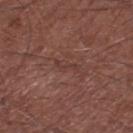This lesion was catalogued during total-body skin photography and was not selected for biopsy.
Cropped from a whole-body photographic skin survey; the tile spans about 15 mm.
This is a white-light tile.
The lesion is located on the leg.
An algorithmic analysis of the crop reported a lesion area of about 2.5 mm² and an outline eccentricity of about 0.95 (0 = round, 1 = elongated). The software also gave an average lesion color of about L≈38 a*≈20 b*≈23 (CIELAB), a lesion–skin lightness drop of about 5, and a lesion-to-skin contrast of about 4.5 (normalized; higher = more distinct). The analysis additionally found a border-irregularity rating of about 7/10 and a within-lesion color-variation index near 0/10.
The subject is a male roughly 65 years of age.